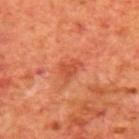workup = no biopsy performed (imaged during a skin exam)
anatomic site = the mid back
imaging modality = total-body-photography crop, ~15 mm field of view
patient = male, aged 68 to 72